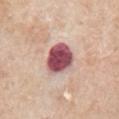follow-up: total-body-photography surveillance lesion; no biopsy | illumination: white-light | patient: male, roughly 65 years of age | automated lesion analysis: a classifier nevus-likeness of about 10/100 and lesion-presence confidence of about 100/100 | lesion diameter: about 4 mm | location: the chest | image source: 15 mm crop, total-body photography.